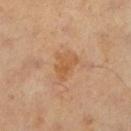follow-up — imaged on a skin check; not biopsied
image-analysis metrics — a footprint of about 7 mm² and a symmetry-axis asymmetry near 0.35; a border-irregularity index near 4/10 and a peripheral color-asymmetry measure near 1; a classifier nevus-likeness of about 0/100 and lesion-presence confidence of about 100/100
tile lighting — cross-polarized illumination
site — the right lower leg
patient — male, approximately 60 years of age
imaging modality — ~15 mm crop, total-body skin-cancer survey